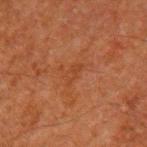Assessment:
Imaged during a routine full-body skin examination; the lesion was not biopsied and no histopathology is available.
Image and clinical context:
The tile uses cross-polarized illumination. A male patient aged around 60. The lesion is located on the left upper arm. Cropped from a whole-body photographic skin survey; the tile spans about 15 mm. The recorded lesion diameter is about 3 mm.